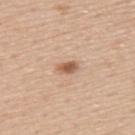Imaged during a routine full-body skin examination; the lesion was not biopsied and no histopathology is available.
A 15 mm close-up extracted from a 3D total-body photography capture.
Automated tile analysis of the lesion measured an area of roughly 3.5 mm² and an outline eccentricity of about 0.8 (0 = round, 1 = elongated). The software also gave a border-irregularity rating of about 2/10, internal color variation of about 3 on a 0–10 scale, and peripheral color asymmetry of about 1.
Located on the upper back.
Captured under white-light illumination.
Measured at roughly 2.5 mm in maximum diameter.
A male patient aged 53–57.